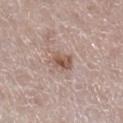Assessment:
The lesion was tiled from a total-body skin photograph and was not biopsied.
Background:
The lesion-visualizer software estimated a footprint of about 5.5 mm², an eccentricity of roughly 0.65, and two-axis asymmetry of about 0.25. It also reported a lesion–skin lightness drop of about 10. And it measured internal color variation of about 5 on a 0–10 scale and a peripheral color-asymmetry measure near 1.5. And it measured a classifier nevus-likeness of about 80/100. The patient is a female aged 48–52. This image is a 15 mm lesion crop taken from a total-body photograph. Longest diameter approximately 3 mm. Captured under white-light illumination. The lesion is on the right lower leg.